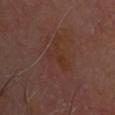Q: Was a biopsy performed?
A: no biopsy performed (imaged during a skin exam)
Q: What kind of image is this?
A: ~15 mm tile from a whole-body skin photo
Q: What lighting was used for the tile?
A: cross-polarized
Q: Automated lesion metrics?
A: a mean CIELAB color near L≈31 a*≈19 b*≈25
Q: What is the lesion's diameter?
A: ~3.5 mm (longest diameter)
Q: Patient demographics?
A: male, aged approximately 65
Q: What is the anatomic site?
A: the head or neck